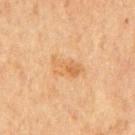lesion size = about 4.5 mm; subject = male, about 65 years old; acquisition = total-body-photography crop, ~15 mm field of view; illumination = cross-polarized; anatomic site = the mid back.